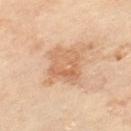{"biopsy_status": "not biopsied; imaged during a skin examination", "lighting": "cross-polarized", "image": {"source": "total-body photography crop", "field_of_view_mm": 15}, "patient": {"sex": "male", "age_approx": 65}, "site": "left thigh", "lesion_size": {"long_diameter_mm_approx": 4.5}}